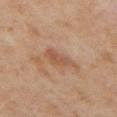No biopsy was performed on this lesion — it was imaged during a full skin examination and was not determined to be concerning.
A 15 mm crop from a total-body photograph taken for skin-cancer surveillance.
The tile uses cross-polarized illumination.
Located on the arm.
Approximately 3.5 mm at its widest.
Automated image analysis of the tile measured a border-irregularity index near 3.5/10 and a peripheral color-asymmetry measure near 1. The software also gave a classifier nevus-likeness of about 0/100.
The patient is a female about 50 years old.